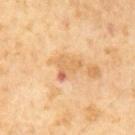biopsy_status: not biopsied; imaged during a skin examination
lighting: cross-polarized
patient:
  sex: male
  age_approx: 65
automated_metrics:
  area_mm2_approx: 6.0
  eccentricity: 0.6
  shape_asymmetry: 0.5
  nevus_likeness_0_100: 0
lesion_size:
  long_diameter_mm_approx: 3.5
image:
  source: total-body photography crop
  field_of_view_mm: 15